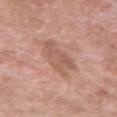This lesion was catalogued during total-body skin photography and was not selected for biopsy.